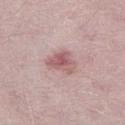  biopsy_status: not biopsied; imaged during a skin examination
  automated_metrics:
    area_mm2_approx: 7.0
    eccentricity: 0.7
    shape_asymmetry: 0.4
    color_variation_0_10: 5.0
    peripheral_color_asymmetry: 1.5
  image:
    source: total-body photography crop
    field_of_view_mm: 15
  lesion_size:
    long_diameter_mm_approx: 4.0
  lighting: white-light
  patient:
    sex: male
    age_approx: 30